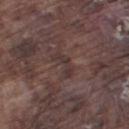| feature | finding |
|---|---|
| follow-up | no biopsy performed (imaged during a skin exam) |
| tile lighting | white-light |
| size | about 3 mm |
| subject | male, about 75 years old |
| site | the leg |
| image-analysis metrics | a lesion area of about 4 mm², a shape eccentricity near 0.85, and a symmetry-axis asymmetry near 0.6; an average lesion color of about L≈33 a*≈15 b*≈17 (CIELAB), a lesion–skin lightness drop of about 6, and a normalized border contrast of about 5.5; border irregularity of about 7 on a 0–10 scale and peripheral color asymmetry of about 0; a classifier nevus-likeness of about 0/100 |
| image source | total-body-photography crop, ~15 mm field of view |